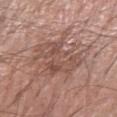Clinical impression: The lesion was tiled from a total-body skin photograph and was not biopsied. Clinical summary: About 6 mm across. Cropped from a total-body skin-imaging series; the visible field is about 15 mm. The lesion is located on the left forearm. A male subject, approximately 70 years of age.